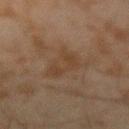Assessment: The lesion was tiled from a total-body skin photograph and was not biopsied. Acquisition and patient details: Cropped from a total-body skin-imaging series; the visible field is about 15 mm. A male subject aged 43 to 47. From the right forearm.